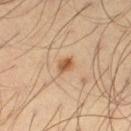Findings:
– notes — no biopsy performed (imaged during a skin exam)
– patient — male, in their mid-40s
– diameter — about 2 mm
– image source — total-body-photography crop, ~15 mm field of view
– lighting — cross-polarized illumination
– anatomic site — the left thigh
– automated metrics — an area of roughly 3 mm², a shape eccentricity near 0.75, and a symmetry-axis asymmetry near 0.35